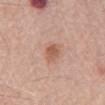biopsy_status: not biopsied; imaged during a skin examination